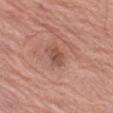Recorded during total-body skin imaging; not selected for excision or biopsy.
From the left thigh.
Automated image analysis of the tile measured a mean CIELAB color near L≈50 a*≈22 b*≈27 and a normalized lesion–skin contrast near 6.5. The software also gave a border-irregularity rating of about 3/10, a within-lesion color-variation index near 2/10, and a peripheral color-asymmetry measure near 0.5.
Imaged with white-light lighting.
The patient is a male aged approximately 80.
A region of skin cropped from a whole-body photographic capture, roughly 15 mm wide.
Longest diameter approximately 3 mm.